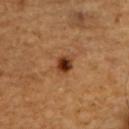Notes:
- notes: imaged on a skin check; not biopsied
- lighting: cross-polarized illumination
- size: about 2.5 mm
- subject: male, roughly 60 years of age
- body site: the back
- automated metrics: a lesion area of about 4 mm² and an outline eccentricity of about 0.55 (0 = round, 1 = elongated); a border-irregularity rating of about 1.5/10 and a peripheral color-asymmetry measure near 1.5
- acquisition: total-body-photography crop, ~15 mm field of view